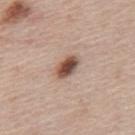Findings:
* workup · catalogued during a skin exam; not biopsied
* subject · male, about 45 years old
* lesion diameter · ~3 mm (longest diameter)
* automated metrics · a lesion area of about 5.5 mm², an eccentricity of roughly 0.8, and a symmetry-axis asymmetry near 0.15; a lesion color around L≈50 a*≈20 b*≈26 in CIELAB, about 17 CIELAB-L* units darker than the surrounding skin, and a lesion-to-skin contrast of about 11.5 (normalized; higher = more distinct); a border-irregularity index near 1.5/10 and radial color variation of about 1.5
* site · the mid back
* imaging modality · ~15 mm tile from a whole-body skin photo